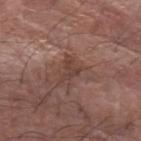Impression: Part of a total-body skin-imaging series; this lesion was reviewed on a skin check and was not flagged for biopsy. Context: The lesion is located on the left forearm. Measured at roughly 3 mm in maximum diameter. The patient is a male approximately 70 years of age. The tile uses white-light illumination. Automated image analysis of the tile measured an area of roughly 5 mm², an outline eccentricity of about 0.7 (0 = round, 1 = elongated), and a symmetry-axis asymmetry near 0.5. A 15 mm crop from a total-body photograph taken for skin-cancer surveillance.